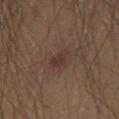{
  "biopsy_status": "not biopsied; imaged during a skin examination",
  "image": {
    "source": "total-body photography crop",
    "field_of_view_mm": 15
  },
  "site": "leg",
  "patient": {
    "sex": "male",
    "age_approx": 70
  }
}